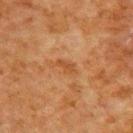Recorded during total-body skin imaging; not selected for excision or biopsy.
The lesion is on the upper back.
A male subject, aged 78 to 82.
A close-up tile cropped from a whole-body skin photograph, about 15 mm across.